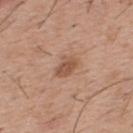– patient · male, aged approximately 40
– anatomic site · the back
– lesion diameter · about 3 mm
– imaging modality · total-body-photography crop, ~15 mm field of view
– illumination · white-light illumination
– automated lesion analysis · an average lesion color of about L≈53 a*≈21 b*≈31 (CIELAB) and a lesion-to-skin contrast of about 7 (normalized; higher = more distinct); border irregularity of about 3 on a 0–10 scale and internal color variation of about 2.5 on a 0–10 scale